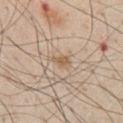Assessment: Recorded during total-body skin imaging; not selected for excision or biopsy. Acquisition and patient details: The lesion is located on the chest. A roughly 15 mm field-of-view crop from a total-body skin photograph. The lesion's longest dimension is about 3 mm. The lesion-visualizer software estimated a footprint of about 3.5 mm², an eccentricity of roughly 0.8, and two-axis asymmetry of about 0.4. The software also gave a mean CIELAB color near L≈60 a*≈15 b*≈32, about 8 CIELAB-L* units darker than the surrounding skin, and a normalized lesion–skin contrast near 6. Imaged with white-light lighting. A male subject aged 58–62.